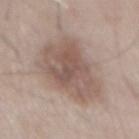<case>
<biopsy_status>not biopsied; imaged during a skin examination</biopsy_status>
<lighting>white-light</lighting>
<image>
  <source>total-body photography crop</source>
  <field_of_view_mm>15</field_of_view_mm>
</image>
<lesion_size>
  <long_diameter_mm_approx>8.0</long_diameter_mm_approx>
</lesion_size>
<site>mid back</site>
<patient>
  <sex>male</sex>
  <age_approx>55</age_approx>
</patient>
<automated_metrics>
  <vs_skin_darker_L>11.0</vs_skin_darker_L>
  <vs_skin_contrast_norm>7.5</vs_skin_contrast_norm>
  <nevus_likeness_0_100>30</nevus_likeness_0_100>
  <lesion_detection_confidence_0_100>100</lesion_detection_confidence_0_100>
</automated_metrics>
</case>